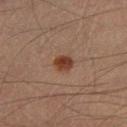notes = catalogued during a skin exam; not biopsied | image source = 15 mm crop, total-body photography | body site = the left thigh | subject = male, about 40 years old | tile lighting = cross-polarized illumination | TBP lesion metrics = about 11 CIELAB-L* units darker than the surrounding skin and a normalized lesion–skin contrast near 10; a border-irregularity rating of about 1/10, a within-lesion color-variation index near 3/10, and radial color variation of about 1; a nevus-likeness score of about 100/100.